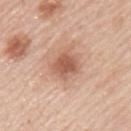Imaged during a routine full-body skin examination; the lesion was not biopsied and no histopathology is available.
A 15 mm close-up extracted from a 3D total-body photography capture.
The lesion is located on the upper back.
The patient is a male roughly 45 years of age.
Captured under white-light illumination.
Approximately 3.5 mm at its widest.